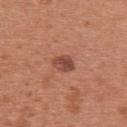Captured during whole-body skin photography for melanoma surveillance; the lesion was not biopsied. Located on the upper back. This is a white-light tile. The subject is a female aged approximately 40. A 15 mm close-up extracted from a 3D total-body photography capture.